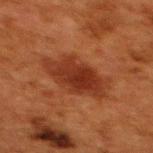Assessment:
Recorded during total-body skin imaging; not selected for excision or biopsy.
Clinical summary:
The total-body-photography lesion software estimated a footprint of about 17 mm². And it measured a lesion color around L≈29 a*≈25 b*≈30 in CIELAB and a lesion-to-skin contrast of about 8.5 (normalized; higher = more distinct). The analysis additionally found border irregularity of about 3 on a 0–10 scale, a within-lesion color-variation index near 3.5/10, and peripheral color asymmetry of about 1. The lesion is located on the upper back. Captured under cross-polarized illumination. A region of skin cropped from a whole-body photographic capture, roughly 15 mm wide. A female subject approximately 50 years of age.